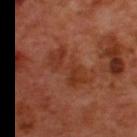workup: total-body-photography surveillance lesion; no biopsy
patient: male, in their 50s
anatomic site: the upper back
acquisition: total-body-photography crop, ~15 mm field of view